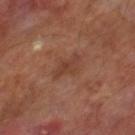biopsy status = no biopsy performed (imaged during a skin exam) | lesion diameter = ≈3.5 mm | patient = male, aged 68 to 72 | image = 15 mm crop, total-body photography | tile lighting = cross-polarized | location = the right lower leg.